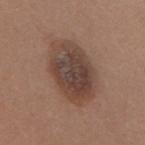Notes:
* biopsy status: catalogued during a skin exam; not biopsied
* lesion diameter: ≈7.5 mm
* location: the upper back
* subject: female, aged approximately 65
* image source: 15 mm crop, total-body photography